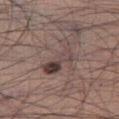Part of a total-body skin-imaging series; this lesion was reviewed on a skin check and was not flagged for biopsy. A male subject roughly 35 years of age. On the right thigh. Cropped from a whole-body photographic skin survey; the tile spans about 15 mm. An algorithmic analysis of the crop reported a shape eccentricity near 0.9. The analysis additionally found an average lesion color of about L≈42 a*≈15 b*≈17 (CIELAB) and about 10 CIELAB-L* units darker than the surrounding skin. The software also gave a border-irregularity rating of about 7/10, internal color variation of about 9 on a 0–10 scale, and a peripheral color-asymmetry measure near 3.5. And it measured a classifier nevus-likeness of about 10/100 and lesion-presence confidence of about 95/100. Measured at roughly 5.5 mm in maximum diameter. Imaged with white-light lighting.